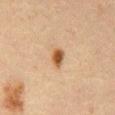<tbp_lesion>
<biopsy_status>not biopsied; imaged during a skin examination</biopsy_status>
<patient>
  <sex>male</sex>
  <age_approx>60</age_approx>
</patient>
<lighting>cross-polarized</lighting>
<site>abdomen</site>
<image>
  <source>total-body photography crop</source>
  <field_of_view_mm>15</field_of_view_mm>
</image>
</tbp_lesion>